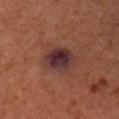biopsy status: no biopsy performed (imaged during a skin exam) | acquisition: total-body-photography crop, ~15 mm field of view | patient: female, in their mid- to late 50s | TBP lesion metrics: roughly 11 lightness units darker than nearby skin and a normalized lesion–skin contrast near 13; a classifier nevus-likeness of about 45/100 and a lesion-detection confidence of about 100/100 | site: the right forearm | tile lighting: cross-polarized illumination | size: about 4 mm.